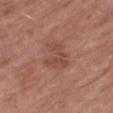notes = imaged on a skin check; not biopsied | TBP lesion metrics = an average lesion color of about L≈48 a*≈22 b*≈27 (CIELAB) and about 7 CIELAB-L* units darker than the surrounding skin; internal color variation of about 3 on a 0–10 scale and a peripheral color-asymmetry measure near 1; an automated nevus-likeness rating near 0 out of 100 and a lesion-detection confidence of about 100/100 | subject = male, in their mid-60s | lesion size = ~4 mm (longest diameter) | location = the leg | image source = ~15 mm tile from a whole-body skin photo.